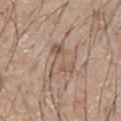Notes:
• notes: imaged on a skin check; not biopsied
• image: ~15 mm tile from a whole-body skin photo
• subject: male, roughly 65 years of age
• lesion diameter: ≈4.5 mm
• anatomic site: the chest
• TBP lesion metrics: border irregularity of about 7 on a 0–10 scale and internal color variation of about 6.5 on a 0–10 scale
• lighting: white-light